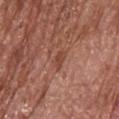Clinical impression:
The lesion was tiled from a total-body skin photograph and was not biopsied.
Image and clinical context:
Imaged with white-light lighting. Automated tile analysis of the lesion measured an outline eccentricity of about 0.85 (0 = round, 1 = elongated) and a symmetry-axis asymmetry near 0.2. The analysis additionally found a lesion color around L≈45 a*≈26 b*≈29 in CIELAB, about 8 CIELAB-L* units darker than the surrounding skin, and a normalized lesion–skin contrast near 6.5. And it measured border irregularity of about 2.5 on a 0–10 scale, a within-lesion color-variation index near 0/10, and radial color variation of about 0. This image is a 15 mm lesion crop taken from a total-body photograph. Located on the head or neck. A male patient, approximately 65 years of age. Measured at roughly 2.5 mm in maximum diameter.